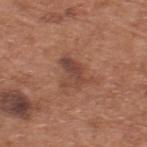Clinical impression: No biopsy was performed on this lesion — it was imaged during a full skin examination and was not determined to be concerning. Background: The subject is a male roughly 65 years of age. A roughly 15 mm field-of-view crop from a total-body skin photograph. From the upper back.